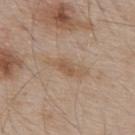workup: total-body-photography surveillance lesion; no biopsy
patient: male, in their mid-50s
body site: the upper back
image: 15 mm crop, total-body photography
tile lighting: white-light
automated metrics: a lesion color around L≈56 a*≈15 b*≈31 in CIELAB, roughly 7 lightness units darker than nearby skin, and a lesion-to-skin contrast of about 6 (normalized; higher = more distinct); a nevus-likeness score of about 0/100